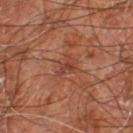Assessment: No biopsy was performed on this lesion — it was imaged during a full skin examination and was not determined to be concerning. Background: A 15 mm close-up tile from a total-body photography series done for melanoma screening. The lesion-visualizer software estimated an eccentricity of roughly 0.8 and a symmetry-axis asymmetry near 0.35. A male subject approximately 60 years of age. The tile uses cross-polarized illumination. The lesion is on the leg. Measured at roughly 3 mm in maximum diameter.